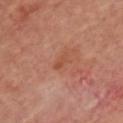Clinical impression:
Imaged during a routine full-body skin examination; the lesion was not biopsied and no histopathology is available.
Background:
The subject is aged 53–57. Cropped from a total-body skin-imaging series; the visible field is about 15 mm. The lesion is on the chest. The recorded lesion diameter is about 2.5 mm. The tile uses cross-polarized illumination.